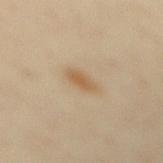Q: Was this lesion biopsied?
A: no biopsy performed (imaged during a skin exam)
Q: Illumination type?
A: cross-polarized illumination
Q: How was this image acquired?
A: ~15 mm tile from a whole-body skin photo
Q: Where on the body is the lesion?
A: the mid back
Q: Automated lesion metrics?
A: a mean CIELAB color near L≈55 a*≈15 b*≈34, a lesion–skin lightness drop of about 9, and a normalized lesion–skin contrast near 7
Q: Patient demographics?
A: female, approximately 35 years of age
Q: What is the lesion's diameter?
A: ≈3 mm